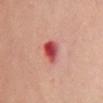Findings:
– notes — no biopsy performed (imaged during a skin exam)
– illumination — cross-polarized
– body site — the front of the torso
– lesion size — about 3 mm
– image source — ~15 mm tile from a whole-body skin photo
– patient — female, aged 58–62